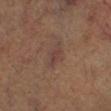Captured during whole-body skin photography for melanoma surveillance; the lesion was not biopsied.
Automated image analysis of the tile measured a lesion color around L≈34 a*≈15 b*≈19 in CIELAB and about 5 CIELAB-L* units darker than the surrounding skin.
The lesion is located on the left lower leg.
The lesion's longest dimension is about 3.5 mm.
This is a cross-polarized tile.
A 15 mm close-up extracted from a 3D total-body photography capture.
A male subject approximately 85 years of age or older.